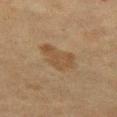No biopsy was performed on this lesion — it was imaged during a full skin examination and was not determined to be concerning. This is a cross-polarized tile. About 4.5 mm across. A region of skin cropped from a whole-body photographic capture, roughly 15 mm wide. Automated image analysis of the tile measured a lesion area of about 9.5 mm². The software also gave a mean CIELAB color near L≈42 a*≈14 b*≈29 and a normalized lesion–skin contrast near 6. It also reported a border-irregularity rating of about 3.5/10, internal color variation of about 1.5 on a 0–10 scale, and radial color variation of about 0.5. The analysis additionally found a nevus-likeness score of about 10/100 and lesion-presence confidence of about 100/100. The lesion is located on the left thigh. A female subject, approximately 40 years of age.